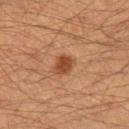The lesion was photographed on a routine skin check and not biopsied; there is no pathology result. The lesion is located on the left forearm. Imaged with cross-polarized lighting. The subject is a male roughly 35 years of age. About 2.5 mm across. An algorithmic analysis of the crop reported a lesion area of about 4.5 mm², a shape eccentricity near 0.7, and a shape-asymmetry score of about 0.3 (0 = symmetric). And it measured a border-irregularity index near 2.5/10, internal color variation of about 2 on a 0–10 scale, and peripheral color asymmetry of about 0.5. The software also gave an automated nevus-likeness rating near 95 out of 100 and lesion-presence confidence of about 100/100. A 15 mm crop from a total-body photograph taken for skin-cancer surveillance.